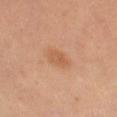Imaged during a routine full-body skin examination; the lesion was not biopsied and no histopathology is available. A lesion tile, about 15 mm wide, cut from a 3D total-body photograph. Imaged with cross-polarized lighting. A female subject, in their 50s. Located on the left thigh.